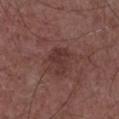| feature | finding |
|---|---|
| biopsy status | catalogued during a skin exam; not biopsied |
| patient | male, in their 60s |
| automated metrics | a lesion area of about 5.5 mm², a shape eccentricity near 0.8, and a shape-asymmetry score of about 0.5 (0 = symmetric); an average lesion color of about L≈33 a*≈20 b*≈20 (CIELAB), a lesion–skin lightness drop of about 7, and a lesion-to-skin contrast of about 6.5 (normalized; higher = more distinct); border irregularity of about 5.5 on a 0–10 scale, a within-lesion color-variation index near 1.5/10, and peripheral color asymmetry of about 0.5 |
| tile lighting | white-light illumination |
| imaging modality | ~15 mm crop, total-body skin-cancer survey |
| location | the chest |